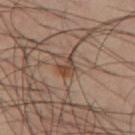Part of a total-body skin-imaging series; this lesion was reviewed on a skin check and was not flagged for biopsy.
Measured at roughly 2.5 mm in maximum diameter.
The total-body-photography lesion software estimated a shape eccentricity near 0.55 and a symmetry-axis asymmetry near 0.4. The software also gave a lesion color around L≈43 a*≈17 b*≈27 in CIELAB and a lesion-to-skin contrast of about 7.5 (normalized; higher = more distinct). And it measured a border-irregularity rating of about 4/10, a color-variation rating of about 4/10, and radial color variation of about 1.5.
From the left thigh.
A 15 mm crop from a total-body photograph taken for skin-cancer surveillance.
A male patient, aged 38–42.
This is a cross-polarized tile.